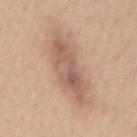Q: Was a biopsy performed?
A: catalogued during a skin exam; not biopsied
Q: How large is the lesion?
A: ≈8 mm
Q: Who is the patient?
A: female, in their mid-40s
Q: Where on the body is the lesion?
A: the mid back
Q: What kind of image is this?
A: 15 mm crop, total-body photography
Q: What lighting was used for the tile?
A: white-light